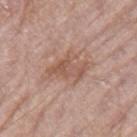No biopsy was performed on this lesion — it was imaged during a full skin examination and was not determined to be concerning.
Imaged with white-light lighting.
The patient is a female aged 73 to 77.
A close-up tile cropped from a whole-body skin photograph, about 15 mm across.
Automated image analysis of the tile measured border irregularity of about 5.5 on a 0–10 scale, a color-variation rating of about 4/10, and peripheral color asymmetry of about 1. It also reported a nevus-likeness score of about 60/100 and a detector confidence of about 100 out of 100 that the crop contains a lesion.
The recorded lesion diameter is about 4.5 mm.
Located on the right thigh.